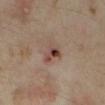Notes:
– workup: imaged on a skin check; not biopsied